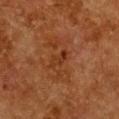Q: Is there a histopathology result?
A: imaged on a skin check; not biopsied
Q: What lighting was used for the tile?
A: cross-polarized illumination
Q: What is the anatomic site?
A: the front of the torso
Q: What is the lesion's diameter?
A: ~3 mm (longest diameter)
Q: What kind of image is this?
A: 15 mm crop, total-body photography
Q: Who is the patient?
A: female, aged 48 to 52